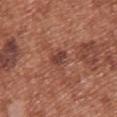Assessment:
Captured during whole-body skin photography for melanoma surveillance; the lesion was not biopsied.
Clinical summary:
Cropped from a total-body skin-imaging series; the visible field is about 15 mm. Automated image analysis of the tile measured internal color variation of about 1 on a 0–10 scale and radial color variation of about 0.5. The software also gave a nevus-likeness score of about 5/100. Located on the back. A female subject aged approximately 40. Captured under white-light illumination.